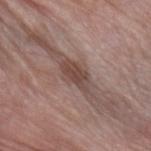Part of a total-body skin-imaging series; this lesion was reviewed on a skin check and was not flagged for biopsy.
A 15 mm close-up tile from a total-body photography series done for melanoma screening.
This is a white-light tile.
A female patient aged around 75.
The lesion is on the arm.